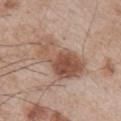workup: catalogued during a skin exam; not biopsied
acquisition: ~15 mm tile from a whole-body skin photo
subject: male, in their mid-60s
lighting: white-light
lesion diameter: ~7 mm (longest diameter)
anatomic site: the arm